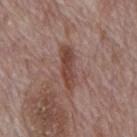biopsy status — no biopsy performed (imaged during a skin exam)
patient — male, roughly 70 years of age
illumination — white-light illumination
body site — the mid back
lesion diameter — ~6 mm (longest diameter)
automated lesion analysis — an area of roughly 9.5 mm², an eccentricity of roughly 0.95, and a shape-asymmetry score of about 0.3 (0 = symmetric); an average lesion color of about L≈45 a*≈20 b*≈24 (CIELAB), roughly 10 lightness units darker than nearby skin, and a normalized lesion–skin contrast near 8; a color-variation rating of about 3.5/10 and a peripheral color-asymmetry measure near 1.5; a classifier nevus-likeness of about 0/100 and lesion-presence confidence of about 100/100
image source — 15 mm crop, total-body photography